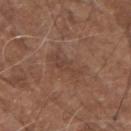Automated tile analysis of the lesion measured a classifier nevus-likeness of about 0/100 and a lesion-detection confidence of about 100/100.
A 15 mm close-up extracted from a 3D total-body photography capture.
A male subject about 75 years old.
From the left forearm.